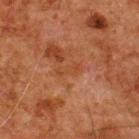No biopsy was performed on this lesion — it was imaged during a full skin examination and was not determined to be concerning.
An algorithmic analysis of the crop reported an area of roughly 3 mm² and an outline eccentricity of about 0.95 (0 = round, 1 = elongated).
The lesion is on the back.
Cropped from a whole-body photographic skin survey; the tile spans about 15 mm.
A male subject aged 78 to 82.
Approximately 3 mm at its widest.
This is a cross-polarized tile.